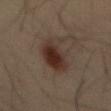Q: Was this lesion biopsied?
A: imaged on a skin check; not biopsied
Q: Patient demographics?
A: male, aged 33–37
Q: What lighting was used for the tile?
A: cross-polarized illumination
Q: How was this image acquired?
A: total-body-photography crop, ~15 mm field of view
Q: Lesion location?
A: the back
Q: Automated lesion metrics?
A: border irregularity of about 4.5 on a 0–10 scale; an automated nevus-likeness rating near 95 out of 100 and lesion-presence confidence of about 100/100
Q: What is the lesion's diameter?
A: about 7 mm